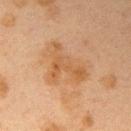Impression:
Part of a total-body skin-imaging series; this lesion was reviewed on a skin check and was not flagged for biopsy.